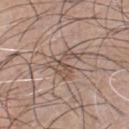<tbp_lesion>
<site>chest</site>
<image>
  <source>total-body photography crop</source>
  <field_of_view_mm>15</field_of_view_mm>
</image>
<patient>
  <sex>male</sex>
  <age_approx>45</age_approx>
</patient>
</tbp_lesion>